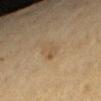Impression:
No biopsy was performed on this lesion — it was imaged during a full skin examination and was not determined to be concerning.
Acquisition and patient details:
A female subject, roughly 50 years of age. A lesion tile, about 15 mm wide, cut from a 3D total-body photograph. On the right forearm.